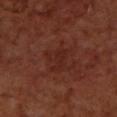The lesion was tiled from a total-body skin photograph and was not biopsied. A close-up tile cropped from a whole-body skin photograph, about 15 mm across. A male subject, approximately 70 years of age. From the upper back. Measured at roughly 4 mm in maximum diameter.